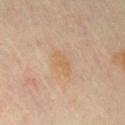The lesion was photographed on a routine skin check and not biopsied; there is no pathology result. Captured under cross-polarized illumination. Measured at roughly 3.5 mm in maximum diameter. A female subject, aged 43–47. A close-up tile cropped from a whole-body skin photograph, about 15 mm across. On the chest.